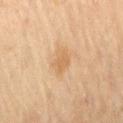Clinical impression: The lesion was tiled from a total-body skin photograph and was not biopsied. Context: A close-up tile cropped from a whole-body skin photograph, about 15 mm across. Measured at roughly 3 mm in maximum diameter. The subject is a male roughly 70 years of age. This is a cross-polarized tile. The lesion is on the back.